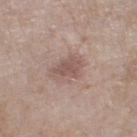<tbp_lesion>
<biopsy_status>not biopsied; imaged during a skin examination</biopsy_status>
<automated_metrics>
  <cielab_L>53</cielab_L>
  <cielab_a>18</cielab_a>
  <cielab_b>22</cielab_b>
  <vs_skin_darker_L>9.0</vs_skin_darker_L>
</automated_metrics>
<image>
  <source>total-body photography crop</source>
  <field_of_view_mm>15</field_of_view_mm>
</image>
<lighting>white-light</lighting>
<site>left thigh</site>
<patient>
  <sex>female</sex>
  <age_approx>75</age_approx>
</patient>
<lesion_size>
  <long_diameter_mm_approx>4.0</long_diameter_mm_approx>
</lesion_size>
</tbp_lesion>